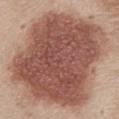Findings:
• notes: no biopsy performed (imaged during a skin exam)
• acquisition: 15 mm crop, total-body photography
• lesion diameter: ~12.5 mm (longest diameter)
• site: the chest
• patient: female, roughly 45 years of age